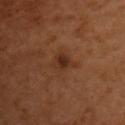{
  "image": {
    "source": "total-body photography crop",
    "field_of_view_mm": 15
  },
  "patient": {
    "sex": "male",
    "age_approx": 50
  },
  "site": "chest",
  "automated_metrics": {
    "area_mm2_approx": 4.5,
    "border_irregularity_0_10": 3.5,
    "peripheral_color_asymmetry": 0.5
  }
}